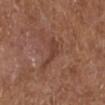Case summary:
– subject: male, in their mid-70s
– body site: the right lower leg
– imaging modality: ~15 mm tile from a whole-body skin photo
– tile lighting: white-light